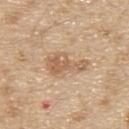The lesion is located on the upper back. Automated image analysis of the tile measured border irregularity of about 5 on a 0–10 scale and a within-lesion color-variation index near 3.5/10. A 15 mm close-up tile from a total-body photography series done for melanoma screening. Approximately 5.5 mm at its widest. A male subject, aged 68–72.